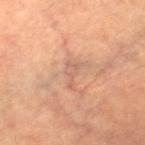Case summary:
– workup — no biopsy performed (imaged during a skin exam)
– automated metrics — a footprint of about 3.5 mm² and an eccentricity of roughly 0.85; a mean CIELAB color near L≈58 a*≈21 b*≈29, about 6 CIELAB-L* units darker than the surrounding skin, and a normalized lesion–skin contrast near 4.5; a border-irregularity rating of about 7/10 and a within-lesion color-variation index near 1.5/10; a nevus-likeness score of about 0/100 and a detector confidence of about 65 out of 100 that the crop contains a lesion
– imaging modality — ~15 mm tile from a whole-body skin photo
– subject — female, aged 63–67
– lesion size — about 3 mm
– site — the right thigh